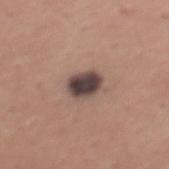{
  "biopsy_status": "not biopsied; imaged during a skin examination",
  "image": {
    "source": "total-body photography crop",
    "field_of_view_mm": 15
  },
  "patient": {
    "sex": "male",
    "age_approx": 45
  },
  "lesion_size": {
    "long_diameter_mm_approx": 3.5
  },
  "site": "back",
  "lighting": "white-light",
  "automated_metrics": {
    "eccentricity": 0.6,
    "shape_asymmetry": 0.1,
    "cielab_L": 42,
    "cielab_a": 14,
    "cielab_b": 17,
    "vs_skin_darker_L": 18.0,
    "lesion_detection_confidence_0_100": 100
  }
}